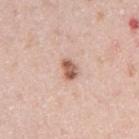Acquisition and patient details: The patient is a male aged 38 to 42. The lesion is on the arm. Automated image analysis of the tile measured a lesion color around L≈60 a*≈21 b*≈29 in CIELAB, a lesion–skin lightness drop of about 14, and a normalized lesion–skin contrast near 9. It also reported a nevus-likeness score of about 90/100 and a lesion-detection confidence of about 100/100. A region of skin cropped from a whole-body photographic capture, roughly 15 mm wide.